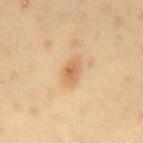{"biopsy_status": "not biopsied; imaged during a skin examination", "automated_metrics": {"eccentricity": 0.75, "cielab_L": 65, "cielab_a": 21, "cielab_b": 39, "vs_skin_contrast_norm": 6.5}, "image": {"source": "total-body photography crop", "field_of_view_mm": 15}, "patient": {"sex": "male", "age_approx": 40}, "site": "mid back", "lighting": "cross-polarized", "lesion_size": {"long_diameter_mm_approx": 2.5}}